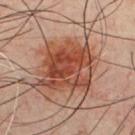notes: imaged on a skin check; not biopsied
patient: male, about 50 years old
image-analysis metrics: an average lesion color of about L≈46 a*≈26 b*≈31 (CIELAB), about 12 CIELAB-L* units darker than the surrounding skin, and a lesion-to-skin contrast of about 9.5 (normalized; higher = more distinct); a border-irregularity index near 3.5/10 and a peripheral color-asymmetry measure near 2; a classifier nevus-likeness of about 95/100
acquisition: ~15 mm tile from a whole-body skin photo
anatomic site: the chest
lesion diameter: ~6 mm (longest diameter)
lighting: cross-polarized